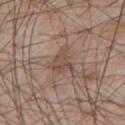The lesion was photographed on a routine skin check and not biopsied; there is no pathology result.
Approximately 3.5 mm at its widest.
Cropped from a total-body skin-imaging series; the visible field is about 15 mm.
A male subject, aged 58 to 62.
Located on the chest.